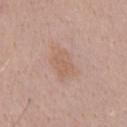Imaged during a routine full-body skin examination; the lesion was not biopsied and no histopathology is available.
Located on the chest.
The total-body-photography lesion software estimated an area of roughly 5 mm², a shape eccentricity near 0.75, and two-axis asymmetry of about 0.25. The software also gave a mean CIELAB color near L≈60 a*≈19 b*≈29, about 6 CIELAB-L* units darker than the surrounding skin, and a normalized lesion–skin contrast near 5. The software also gave a border-irregularity index near 2.5/10, internal color variation of about 2 on a 0–10 scale, and radial color variation of about 0.5.
The tile uses white-light illumination.
Cropped from a whole-body photographic skin survey; the tile spans about 15 mm.
Longest diameter approximately 3 mm.
A male subject roughly 55 years of age.